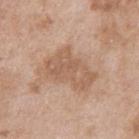Captured during whole-body skin photography for melanoma surveillance; the lesion was not biopsied. The recorded lesion diameter is about 5.5 mm. The lesion is located on the right upper arm. A region of skin cropped from a whole-body photographic capture, roughly 15 mm wide. Automated tile analysis of the lesion measured a lesion area of about 16 mm² and a symmetry-axis asymmetry near 0.35. The analysis additionally found a lesion color around L≈58 a*≈18 b*≈31 in CIELAB and roughly 8 lightness units darker than nearby skin. It also reported a color-variation rating of about 3/10 and radial color variation of about 1. The software also gave a lesion-detection confidence of about 100/100. A male subject about 50 years old.